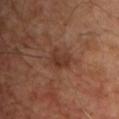<lesion>
<biopsy_status>not biopsied; imaged during a skin examination</biopsy_status>
<lesion_size>
  <long_diameter_mm_approx>3.0</long_diameter_mm_approx>
</lesion_size>
<site>chest</site>
<image>
  <source>total-body photography crop</source>
  <field_of_view_mm>15</field_of_view_mm>
</image>
<lighting>cross-polarized</lighting>
<automated_metrics>
  <vs_skin_contrast_norm>6.5</vs_skin_contrast_norm>
  <nevus_likeness_0_100>5</nevus_likeness_0_100>
  <lesion_detection_confidence_0_100>100</lesion_detection_confidence_0_100>
</automated_metrics>
<patient>
  <sex>male</sex>
  <age_approx>55</age_approx>
</patient>
</lesion>